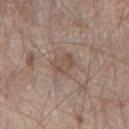Findings:
• site: the chest
• patient: male, roughly 80 years of age
• image: 15 mm crop, total-body photography
• lesion size: ~2.5 mm (longest diameter)
• tile lighting: white-light